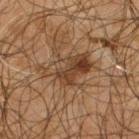Imaged during a routine full-body skin examination; the lesion was not biopsied and no histopathology is available. A 15 mm close-up extracted from a 3D total-body photography capture. Imaged with cross-polarized lighting. An algorithmic analysis of the crop reported a shape eccentricity near 0.8 and a symmetry-axis asymmetry near 0.35. The software also gave a border-irregularity index near 6/10 and a color-variation rating of about 8.5/10. It also reported lesion-presence confidence of about 90/100. The subject is a male in their mid- to late 60s. The lesion is on the upper back.